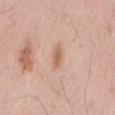Assessment:
Captured during whole-body skin photography for melanoma surveillance; the lesion was not biopsied.
Background:
A male subject, aged 53–57. The lesion is on the back. A 15 mm close-up tile from a total-body photography series done for melanoma screening.